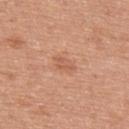Clinical impression:
No biopsy was performed on this lesion — it was imaged during a full skin examination and was not determined to be concerning.
Image and clinical context:
A male patient aged 53 to 57. A region of skin cropped from a whole-body photographic capture, roughly 15 mm wide. From the upper back.